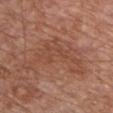Clinical summary: The tile uses white-light illumination. Measured at roughly 8.5 mm in maximum diameter. Cropped from a whole-body photographic skin survey; the tile spans about 15 mm. Located on the chest. Automated tile analysis of the lesion measured an eccentricity of roughly 0.8 and a symmetry-axis asymmetry near 0.65. It also reported a mean CIELAB color near L≈47 a*≈23 b*≈29 and roughly 6 lightness units darker than nearby skin. The analysis additionally found border irregularity of about 10 on a 0–10 scale, a color-variation rating of about 2.5/10, and radial color variation of about 1. A male subject roughly 65 years of age.